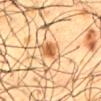Notes:
• workup: no biopsy performed (imaged during a skin exam)
• subject: male, in their 60s
• lesion diameter: about 3 mm
• lighting: cross-polarized
• automated lesion analysis: a mean CIELAB color near L≈49 a*≈19 b*≈34 and roughly 11 lightness units darker than nearby skin
• imaging modality: total-body-photography crop, ~15 mm field of view
• anatomic site: the back